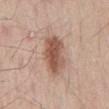Clinical impression:
Captured during whole-body skin photography for melanoma surveillance; the lesion was not biopsied.
Image and clinical context:
This is a white-light tile. A male subject, approximately 30 years of age. A lesion tile, about 15 mm wide, cut from a 3D total-body photograph. The lesion is on the lower back.